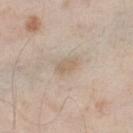notes=catalogued during a skin exam; not biopsied
imaging modality=~15 mm crop, total-body skin-cancer survey
body site=the leg
patient=male, approximately 60 years of age
illumination=white-light illumination